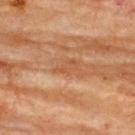Assessment: The lesion was photographed on a routine skin check and not biopsied; there is no pathology result. Context: Located on the upper back. Cropped from a whole-body photographic skin survey; the tile spans about 15 mm. Captured under cross-polarized illumination. Approximately 3 mm at its widest. The subject is a female approximately 80 years of age.